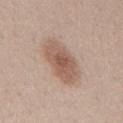• notes — catalogued during a skin exam; not biopsied
• illumination — white-light
• body site — the mid back
• size — about 6 mm
• automated lesion analysis — a shape eccentricity near 0.85 and a symmetry-axis asymmetry near 0.15; a lesion color around L≈57 a*≈18 b*≈27 in CIELAB, roughly 11 lightness units darker than nearby skin, and a lesion-to-skin contrast of about 7.5 (normalized; higher = more distinct)
• acquisition — 15 mm crop, total-body photography
• patient — male, about 40 years old